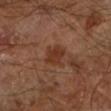notes=total-body-photography surveillance lesion; no biopsy
image source=~15 mm tile from a whole-body skin photo
location=the leg
diameter=~3 mm (longest diameter)
tile lighting=cross-polarized illumination
patient=aged 63–67
image-analysis metrics=an area of roughly 5.5 mm², an eccentricity of roughly 0.7, and a symmetry-axis asymmetry near 0.3; a within-lesion color-variation index near 2/10 and peripheral color asymmetry of about 0.5